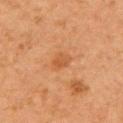Recorded during total-body skin imaging; not selected for excision or biopsy. The total-body-photography lesion software estimated an average lesion color of about L≈46 a*≈23 b*≈35 (CIELAB) and a normalized border contrast of about 5.5. The software also gave a border-irregularity index near 2/10 and a color-variation rating of about 2/10. It also reported a classifier nevus-likeness of about 5/100 and lesion-presence confidence of about 100/100. The lesion is located on the left upper arm. A 15 mm close-up tile from a total-body photography series done for melanoma screening. Approximately 2.5 mm at its widest. The patient is a male roughly 65 years of age.